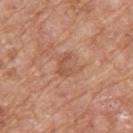No biopsy was performed on this lesion — it was imaged during a full skin examination and was not determined to be concerning.